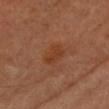No biopsy was performed on this lesion — it was imaged during a full skin examination and was not determined to be concerning.
An algorithmic analysis of the crop reported about 6 CIELAB-L* units darker than the surrounding skin and a normalized lesion–skin contrast near 6.5.
A female patient, about 60 years old.
On the head or neck.
Cropped from a total-body skin-imaging series; the visible field is about 15 mm.
Longest diameter approximately 3 mm.